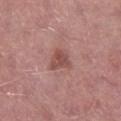Background: This image is a 15 mm lesion crop taken from a total-body photograph. The total-body-photography lesion software estimated an average lesion color of about L≈49 a*≈24 b*≈23 (CIELAB), a lesion–skin lightness drop of about 10, and a normalized lesion–skin contrast near 7. And it measured an automated nevus-likeness rating near 15 out of 100 and lesion-presence confidence of about 100/100. Imaged with white-light lighting. The lesion is located on the right lower leg. The patient is a male in their mid-50s. About 3 mm across.